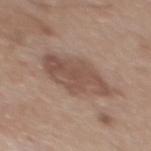Longest diameter approximately 7.5 mm. Captured under white-light illumination. A male subject, about 65 years old. From the back. The total-body-photography lesion software estimated a lesion area of about 22 mm², an eccentricity of roughly 0.85, and a shape-asymmetry score of about 0.2 (0 = symmetric). It also reported an average lesion color of about L≈51 a*≈17 b*≈25 (CIELAB) and a lesion–skin lightness drop of about 10. The software also gave a border-irregularity index near 3/10 and a peripheral color-asymmetry measure near 1. A 15 mm close-up tile from a total-body photography series done for melanoma screening.